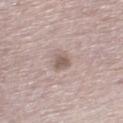image source — ~15 mm crop, total-body skin-cancer survey; subject — male, in their mid- to late 70s; anatomic site — the leg.